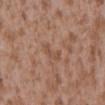Impression: This lesion was catalogued during total-body skin photography and was not selected for biopsy. Context: A male patient aged 43–47. A close-up tile cropped from a whole-body skin photograph, about 15 mm across. From the mid back. Measured at roughly 3 mm in maximum diameter. Automated tile analysis of the lesion measured an area of roughly 3.5 mm², a shape eccentricity near 0.8, and two-axis asymmetry of about 0.45. And it measured an average lesion color of about L≈50 a*≈20 b*≈29 (CIELAB), a lesion–skin lightness drop of about 6, and a normalized lesion–skin contrast near 5. The analysis additionally found an automated nevus-likeness rating near 0 out of 100 and lesion-presence confidence of about 100/100.